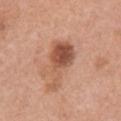Imaged during a routine full-body skin examination; the lesion was not biopsied and no histopathology is available.
On the right upper arm.
Measured at roughly 6.5 mm in maximum diameter.
The lesion-visualizer software estimated a lesion-detection confidence of about 100/100.
A female subject approximately 60 years of age.
Cropped from a whole-body photographic skin survey; the tile spans about 15 mm.
Imaged with white-light lighting.